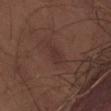Assessment: The lesion was photographed on a routine skin check and not biopsied; there is no pathology result. Clinical summary: Automated image analysis of the tile measured an average lesion color of about L≈28 a*≈17 b*≈19 (CIELAB), about 5 CIELAB-L* units darker than the surrounding skin, and a normalized lesion–skin contrast near 5.5. The software also gave a lesion-detection confidence of about 95/100. The patient is a male aged 58 to 62. Located on the leg. The tile uses cross-polarized illumination. The lesion's longest dimension is about 2.5 mm. This image is a 15 mm lesion crop taken from a total-body photograph.